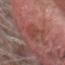location=the head or neck | lighting=white-light | patient=male, in their mid- to late 70s | imaging modality=total-body-photography crop, ~15 mm field of view | size=≈4 mm.